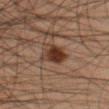Case summary:
- biopsy status — no biopsy performed (imaged during a skin exam)
- lesion diameter — about 3.5 mm
- patient — male, aged 43–47
- location — the leg
- image — 15 mm crop, total-body photography